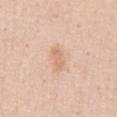Background:
An algorithmic analysis of the crop reported border irregularity of about 2.5 on a 0–10 scale, a within-lesion color-variation index near 1/10, and a peripheral color-asymmetry measure near 0.5. The analysis additionally found a nevus-likeness score of about 10/100 and lesion-presence confidence of about 100/100. A male patient aged 48 to 52. This is a white-light tile. A 15 mm close-up tile from a total-body photography series done for melanoma screening. Longest diameter approximately 3 mm. From the front of the torso.